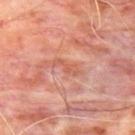Recorded during total-body skin imaging; not selected for excision or biopsy. The lesion is on the upper back. A region of skin cropped from a whole-body photographic capture, roughly 15 mm wide. A male patient, in their mid- to late 60s. Automated image analysis of the tile measured an average lesion color of about L≈56 a*≈28 b*≈31 (CIELAB), a lesion–skin lightness drop of about 7, and a normalized lesion–skin contrast near 5. The tile uses cross-polarized illumination. Approximately 3 mm at its widest.